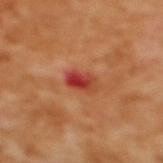notes: catalogued during a skin exam; not biopsied
lesion size: ~3 mm (longest diameter)
automated metrics: a border-irregularity rating of about 2/10, a color-variation rating of about 9.5/10, and radial color variation of about 3.5; an automated nevus-likeness rating near 0 out of 100 and lesion-presence confidence of about 100/100
body site: the upper back
tile lighting: cross-polarized illumination
subject: female, aged approximately 55
image: ~15 mm crop, total-body skin-cancer survey